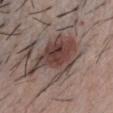<lesion>
<automated_metrics>
  <vs_skin_darker_L>12.0</vs_skin_darker_L>
  <vs_skin_contrast_norm>9.5</vs_skin_contrast_norm>
  <border_irregularity_0_10>6.0</border_irregularity_0_10>
  <color_variation_0_10>6.0</color_variation_0_10>
  <peripheral_color_asymmetry>2.0</peripheral_color_asymmetry>
</automated_metrics>
<image>
  <source>total-body photography crop</source>
  <field_of_view_mm>15</field_of_view_mm>
</image>
<patient>
  <sex>male</sex>
  <age_approx>40</age_approx>
</patient>
<site>chest</site>
<lesion_size>
  <long_diameter_mm_approx>8.0</long_diameter_mm_approx>
</lesion_size>
<lighting>white-light</lighting>
</lesion>